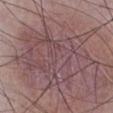Clinical impression: The lesion was tiled from a total-body skin photograph and was not biopsied. Image and clinical context: A region of skin cropped from a whole-body photographic capture, roughly 15 mm wide. The patient is a male aged around 50. Located on the right lower leg.